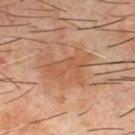Q: Was this lesion biopsied?
A: imaged on a skin check; not biopsied
Q: How was this image acquired?
A: 15 mm crop, total-body photography
Q: Who is the patient?
A: male, in their 60s
Q: What is the lesion's diameter?
A: about 6 mm
Q: Illumination type?
A: cross-polarized
Q: What is the anatomic site?
A: the chest